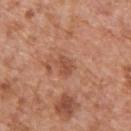follow-up = catalogued during a skin exam; not biopsied
location = the upper back
illumination = white-light
automated metrics = a lesion area of about 3.5 mm² and a shape eccentricity near 0.65
lesion size = about 2.5 mm
image source = ~15 mm tile from a whole-body skin photo
subject = male, approximately 65 years of age